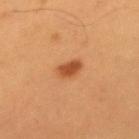This lesion was catalogued during total-body skin photography and was not selected for biopsy.
A male patient aged approximately 55.
The lesion is located on the mid back.
This image is a 15 mm lesion crop taken from a total-body photograph.